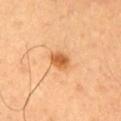  biopsy_status: not biopsied; imaged during a skin examination
  lesion_size:
    long_diameter_mm_approx: 2.5
  site: leg
  image:
    source: total-body photography crop
    field_of_view_mm: 15
  patient:
    sex: male
    age_approx: 50
  lighting: cross-polarized